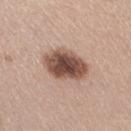This lesion was catalogued during total-body skin photography and was not selected for biopsy. The lesion is on the right thigh. A 15 mm close-up tile from a total-body photography series done for melanoma screening. The tile uses white-light illumination. Automated image analysis of the tile measured a lesion area of about 15 mm² and a symmetry-axis asymmetry near 0.15. Measured at roughly 5 mm in maximum diameter. A female patient, aged approximately 40.